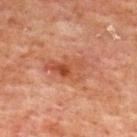workup=total-body-photography surveillance lesion; no biopsy
image=15 mm crop, total-body photography
image-analysis metrics=a lesion–skin lightness drop of about 7 and a normalized lesion–skin contrast near 6.5; a border-irregularity rating of about 5/10, a within-lesion color-variation index near 6.5/10, and peripheral color asymmetry of about 2.5; an automated nevus-likeness rating near 35 out of 100 and a lesion-detection confidence of about 100/100
anatomic site=the upper back
subject=male, about 60 years old
lesion diameter=about 5 mm
illumination=cross-polarized